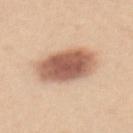This is a white-light tile.
The patient is a female aged 23 to 27.
The lesion's longest dimension is about 6.5 mm.
Automated tile analysis of the lesion measured a lesion color around L≈59 a*≈21 b*≈30 in CIELAB and a lesion-to-skin contrast of about 10.5 (normalized; higher = more distinct).
The lesion is on the back.
A 15 mm close-up extracted from a 3D total-body photography capture.